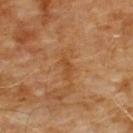Part of a total-body skin-imaging series; this lesion was reviewed on a skin check and was not flagged for biopsy. The lesion's longest dimension is about 2.5 mm. On the chest. Captured under cross-polarized illumination. Cropped from a total-body skin-imaging series; the visible field is about 15 mm. A male patient, aged around 60.